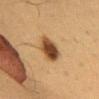follow-up: catalogued during a skin exam; not biopsied
body site: the chest
automated metrics: an average lesion color of about L≈38 a*≈17 b*≈31 (CIELAB), roughly 16 lightness units darker than nearby skin, and a normalized border contrast of about 12.5; a classifier nevus-likeness of about 100/100 and a detector confidence of about 100 out of 100 that the crop contains a lesion
patient: female, aged 38 to 42
lesion diameter: ~3.5 mm (longest diameter)
illumination: cross-polarized
acquisition: 15 mm crop, total-body photography